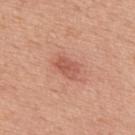biopsy status: total-body-photography surveillance lesion; no biopsy
image-analysis metrics: a footprint of about 5.5 mm², a shape eccentricity near 0.75, and a shape-asymmetry score of about 0.25 (0 = symmetric)
subject: male, aged 48–52
lesion diameter: ~3 mm (longest diameter)
location: the upper back
imaging modality: total-body-photography crop, ~15 mm field of view
illumination: white-light